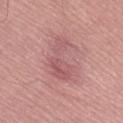{
  "image": {
    "source": "total-body photography crop",
    "field_of_view_mm": 15
  },
  "site": "lower back",
  "patient": {
    "sex": "male",
    "age_approx": 65
  },
  "automated_metrics": {
    "area_mm2_approx": 11.0,
    "shape_asymmetry": 0.6,
    "cielab_L": 56,
    "cielab_a": 26,
    "cielab_b": 20,
    "vs_skin_darker_L": 8.0,
    "vs_skin_contrast_norm": 5.5,
    "border_irregularity_0_10": 7.0,
    "color_variation_0_10": 3.5,
    "peripheral_color_asymmetry": 1.0,
    "nevus_likeness_0_100": 0,
    "lesion_detection_confidence_0_100": 100
  },
  "lighting": "white-light",
  "lesion_size": {
    "long_diameter_mm_approx": 5.5
  }
}